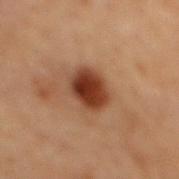Case summary:
* follow-up · catalogued during a skin exam; not biopsied
* location · the mid back
* subject · male, aged around 70
* image · ~15 mm tile from a whole-body skin photo